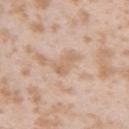Part of a total-body skin-imaging series; this lesion was reviewed on a skin check and was not flagged for biopsy. A region of skin cropped from a whole-body photographic capture, roughly 15 mm wide. The tile uses white-light illumination. A female subject, in their mid- to late 20s. About 3.5 mm across. On the left upper arm. Automated image analysis of the tile measured an area of roughly 4 mm² and two-axis asymmetry of about 0.45.